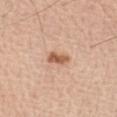The lesion was tiled from a total-body skin photograph and was not biopsied. The lesion is located on the left forearm. A close-up tile cropped from a whole-body skin photograph, about 15 mm across. A male subject, approximately 60 years of age.